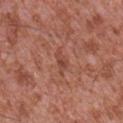Findings:
- biopsy status — no biopsy performed (imaged during a skin exam)
- patient — male, in their mid- to late 40s
- body site — the chest
- acquisition — 15 mm crop, total-body photography
- tile lighting — white-light